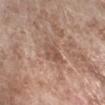Imaged during a routine full-body skin examination; the lesion was not biopsied and no histopathology is available.
Captured under white-light illumination.
The subject is a female aged around 75.
A lesion tile, about 15 mm wide, cut from a 3D total-body photograph.
An algorithmic analysis of the crop reported an area of roughly 5 mm², an outline eccentricity of about 0.5 (0 = round, 1 = elongated), and a symmetry-axis asymmetry near 0.3. And it measured a border-irregularity rating of about 3/10 and a color-variation rating of about 3.5/10.
The lesion is located on the right forearm.
The lesion's longest dimension is about 2.5 mm.